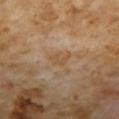The lesion was photographed on a routine skin check and not biopsied; there is no pathology result.
The lesion is located on the chest.
Imaged with cross-polarized lighting.
The patient is a male roughly 60 years of age.
A close-up tile cropped from a whole-body skin photograph, about 15 mm across.